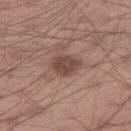Impression: The lesion was photographed on a routine skin check and not biopsied; there is no pathology result. Image and clinical context: From the leg. Imaged with white-light lighting. A region of skin cropped from a whole-body photographic capture, roughly 15 mm wide. Approximately 3.5 mm at its widest. The total-body-photography lesion software estimated a shape-asymmetry score of about 0.2 (0 = symmetric). And it measured a border-irregularity index near 2/10, a within-lesion color-variation index near 3/10, and radial color variation of about 1. The analysis additionally found a nevus-likeness score of about 65/100 and a lesion-detection confidence of about 100/100. A male patient in their 30s.